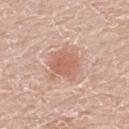biopsy_status: not biopsied; imaged during a skin examination
image:
  source: total-body photography crop
  field_of_view_mm: 15
site: back
lighting: white-light
patient:
  sex: male
  age_approx: 60
lesion_size:
  long_diameter_mm_approx: 3.5